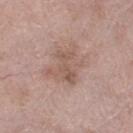workup = catalogued during a skin exam; not biopsied | image source = ~15 mm crop, total-body skin-cancer survey | site = the right lower leg | subject = female, aged 73–77 | lesion size = ~4 mm (longest diameter).